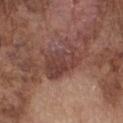The lesion was tiled from a total-body skin photograph and was not biopsied. The lesion is on the chest. Approximately 5 mm at its widest. A male patient aged around 75. This image is a 15 mm lesion crop taken from a total-body photograph.